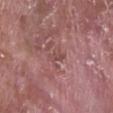{"patient": {"sex": "male", "age_approx": 70}, "site": "right lower leg", "image": {"source": "total-body photography crop", "field_of_view_mm": 15}}